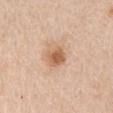notes: catalogued during a skin exam; not biopsied
imaging modality: ~15 mm crop, total-body skin-cancer survey
illumination: white-light illumination
patient: male, aged around 60
lesion diameter: ≈3 mm
site: the right upper arm
automated lesion analysis: a lesion area of about 5.5 mm², an outline eccentricity of about 0.55 (0 = round, 1 = elongated), and a symmetry-axis asymmetry near 0.2; a lesion color around L≈61 a*≈21 b*≈34 in CIELAB and about 12 CIELAB-L* units darker than the surrounding skin; a border-irregularity index near 2/10 and radial color variation of about 1; a nevus-likeness score of about 70/100 and lesion-presence confidence of about 100/100